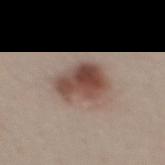biopsy_status: not biopsied; imaged during a skin examination
image:
  source: total-body photography crop
  field_of_view_mm: 15
site: mid back
lighting: white-light
patient:
  sex: male
  age_approx: 30
lesion_size:
  long_diameter_mm_approx: 5.0